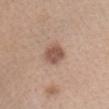Assessment: Part of a total-body skin-imaging series; this lesion was reviewed on a skin check and was not flagged for biopsy. Context: Cropped from a whole-body photographic skin survey; the tile spans about 15 mm. A female subject, approximately 40 years of age. The lesion is on the head or neck. Automated tile analysis of the lesion measured a border-irregularity rating of about 1.5/10, internal color variation of about 2 on a 0–10 scale, and a peripheral color-asymmetry measure near 0.5. Imaged with white-light lighting.